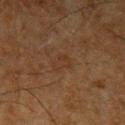Impression:
No biopsy was performed on this lesion — it was imaged during a full skin examination and was not determined to be concerning.
Acquisition and patient details:
The subject is a male approximately 65 years of age. The tile uses cross-polarized illumination. The lesion-visualizer software estimated a lesion area of about 3.5 mm² and an outline eccentricity of about 0.8 (0 = round, 1 = elongated). It also reported a mean CIELAB color near L≈27 a*≈15 b*≈25 and a lesion-to-skin contrast of about 4.5 (normalized; higher = more distinct). It also reported a classifier nevus-likeness of about 0/100 and a lesion-detection confidence of about 100/100. About 2.5 mm across. A 15 mm crop from a total-body photograph taken for skin-cancer surveillance. The lesion is on the left upper arm.